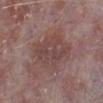Clinical impression: Part of a total-body skin-imaging series; this lesion was reviewed on a skin check and was not flagged for biopsy. Context: From the left lower leg. The recorded lesion diameter is about 6 mm. The patient is a male about 75 years old. An algorithmic analysis of the crop reported a lesion area of about 19 mm², an outline eccentricity of about 0.7 (0 = round, 1 = elongated), and a symmetry-axis asymmetry near 0.25. Cropped from a total-body skin-imaging series; the visible field is about 15 mm.